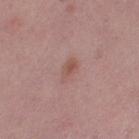biopsy status=catalogued during a skin exam; not biopsied
patient=female, aged around 40
anatomic site=the right thigh
image=~15 mm tile from a whole-body skin photo
diameter=~3 mm (longest diameter)